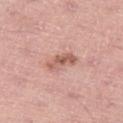Notes:
- biopsy status: imaged on a skin check; not biopsied
- subject: male, aged around 60
- acquisition: 15 mm crop, total-body photography
- tile lighting: white-light illumination
- automated metrics: a detector confidence of about 100 out of 100 that the crop contains a lesion
- size: about 4 mm
- anatomic site: the left thigh